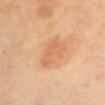Case summary:
• biopsy status: catalogued during a skin exam; not biopsied
• patient: female, roughly 50 years of age
• imaging modality: 15 mm crop, total-body photography
• lesion diameter: ~5 mm (longest diameter)
• site: the chest
• automated lesion analysis: a color-variation rating of about 2/10 and radial color variation of about 0.5
• tile lighting: cross-polarized illumination